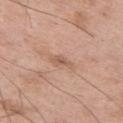The lesion is on the left thigh. A male patient, roughly 50 years of age. A 15 mm close-up extracted from a 3D total-body photography capture.